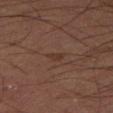follow-up = no biopsy performed (imaged during a skin exam); tile lighting = cross-polarized illumination; automated lesion analysis = a classifier nevus-likeness of about 5/100; diameter = ≈1.5 mm; acquisition = total-body-photography crop, ~15 mm field of view; patient = male, aged 58–62; anatomic site = the right thigh.